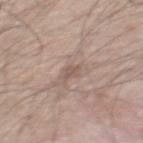Background:
A male subject roughly 50 years of age. A 15 mm close-up tile from a total-body photography series done for melanoma screening. This is a white-light tile. Approximately 2 mm at its widest. An algorithmic analysis of the crop reported a lesion area of about 2 mm², a shape eccentricity near 0.65, and a shape-asymmetry score of about 0.35 (0 = symmetric). And it measured a border-irregularity index near 3.5/10, a within-lesion color-variation index near 0.5/10, and peripheral color asymmetry of about 0. And it measured an automated nevus-likeness rating near 0 out of 100 and lesion-presence confidence of about 95/100. The lesion is located on the back.